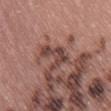notes — no biopsy performed (imaged during a skin exam); patient — female, in their 60s; lighting — white-light; body site — the right thigh; image — 15 mm crop, total-body photography.